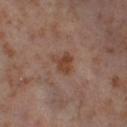diameter: ~3.5 mm (longest diameter) | image source: 15 mm crop, total-body photography | body site: the leg | automated metrics: a lesion area of about 5.5 mm² and a shape-asymmetry score of about 0.4 (0 = symmetric); about 8 CIELAB-L* units darker than the surrounding skin; border irregularity of about 4 on a 0–10 scale, a within-lesion color-variation index near 3/10, and peripheral color asymmetry of about 1 | patient: female, aged around 55.